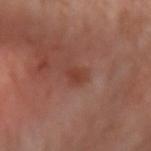{
  "biopsy_status": "not biopsied; imaged during a skin examination",
  "automated_metrics": {
    "eccentricity": 0.8,
    "vs_skin_darker_L": 7.0,
    "vs_skin_contrast_norm": 6.5,
    "border_irregularity_0_10": 2.5,
    "color_variation_0_10": 1.0,
    "peripheral_color_asymmetry": 0.5,
    "nevus_likeness_0_100": 10,
    "lesion_detection_confidence_0_100": 100
  },
  "site": "left forearm",
  "patient": {
    "sex": "male",
    "age_approx": 65
  },
  "lighting": "cross-polarized",
  "image": {
    "source": "total-body photography crop",
    "field_of_view_mm": 15
  }
}